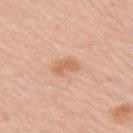{"biopsy_status": "not biopsied; imaged during a skin examination", "patient": {"sex": "female", "age_approx": 50}, "image": {"source": "total-body photography crop", "field_of_view_mm": 15}, "automated_metrics": {"area_mm2_approx": 4.5, "shape_asymmetry": 0.25, "border_irregularity_0_10": 3.0, "color_variation_0_10": 2.0, "peripheral_color_asymmetry": 1.0}, "site": "right upper arm", "lighting": "white-light"}